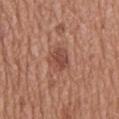Recorded during total-body skin imaging; not selected for excision or biopsy.
Longest diameter approximately 3 mm.
From the back.
An algorithmic analysis of the crop reported an eccentricity of roughly 0.6 and a symmetry-axis asymmetry near 0.2.
This is a white-light tile.
A region of skin cropped from a whole-body photographic capture, roughly 15 mm wide.
The subject is a male roughly 65 years of age.